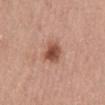Q: Was this lesion biopsied?
A: no biopsy performed (imaged during a skin exam)
Q: What kind of image is this?
A: ~15 mm crop, total-body skin-cancer survey
Q: Lesion location?
A: the abdomen
Q: What did automated image analysis measure?
A: a footprint of about 6 mm² and two-axis asymmetry of about 0.2; internal color variation of about 4 on a 0–10 scale; a classifier nevus-likeness of about 95/100 and a detector confidence of about 100 out of 100 that the crop contains a lesion
Q: Patient demographics?
A: female, aged approximately 60